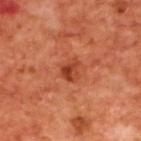<case>
<biopsy_status>not biopsied; imaged during a skin examination</biopsy_status>
<site>upper back</site>
<lesion_size>
  <long_diameter_mm_approx>2.5</long_diameter_mm_approx>
</lesion_size>
<image>
  <source>total-body photography crop</source>
  <field_of_view_mm>15</field_of_view_mm>
</image>
<lighting>cross-polarized</lighting>
<patient>
  <sex>male</sex>
  <age_approx>70</age_approx>
</patient>
</case>